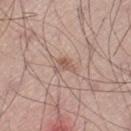<lesion>
  <biopsy_status>not biopsied; imaged during a skin examination</biopsy_status>
  <site>left thigh</site>
  <lighting>white-light</lighting>
  <patient>
    <sex>male</sex>
    <age_approx>45</age_approx>
  </patient>
  <lesion_size>
    <long_diameter_mm_approx>2.5</long_diameter_mm_approx>
  </lesion_size>
  <image>
    <source>total-body photography crop</source>
    <field_of_view_mm>15</field_of_view_mm>
  </image>
</lesion>